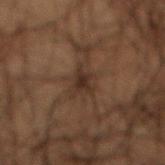A male patient, aged 48–52.
This is a cross-polarized tile.
From the mid back.
A lesion tile, about 15 mm wide, cut from a 3D total-body photograph.
Automated tile analysis of the lesion measured a lesion area of about 4 mm² and a shape eccentricity near 0.8. It also reported an average lesion color of about L≈21 a*≈12 b*≈18 (CIELAB), about 7 CIELAB-L* units darker than the surrounding skin, and a normalized border contrast of about 8.5. The analysis additionally found border irregularity of about 4 on a 0–10 scale, a within-lesion color-variation index near 1.5/10, and radial color variation of about 0.5. And it measured a classifier nevus-likeness of about 0/100 and lesion-presence confidence of about 60/100.
Longest diameter approximately 3 mm.